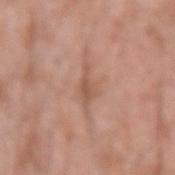Impression:
Imaged during a routine full-body skin examination; the lesion was not biopsied and no histopathology is available.
Background:
From the left forearm. The total-body-photography lesion software estimated a lesion area of about 4 mm², a shape eccentricity near 0.9, and a symmetry-axis asymmetry near 0.45. The software also gave border irregularity of about 5 on a 0–10 scale, internal color variation of about 2 on a 0–10 scale, and a peripheral color-asymmetry measure near 0.5. The software also gave a classifier nevus-likeness of about 0/100. A male patient, roughly 60 years of age. Captured under white-light illumination. A 15 mm close-up extracted from a 3D total-body photography capture. Approximately 4 mm at its widest.